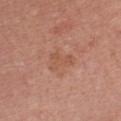Part of a total-body skin-imaging series; this lesion was reviewed on a skin check and was not flagged for biopsy.
From the chest.
A roughly 15 mm field-of-view crop from a total-body skin photograph.
A female patient, roughly 25 years of age.
The lesion's longest dimension is about 3 mm.
Imaged with white-light lighting.